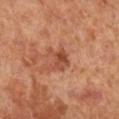Q: Is there a histopathology result?
A: no biopsy performed (imaged during a skin exam)
Q: Automated lesion metrics?
A: a footprint of about 5.5 mm², an eccentricity of roughly 0.5, and a shape-asymmetry score of about 0.45 (0 = symmetric); a nevus-likeness score of about 40/100 and a lesion-detection confidence of about 100/100
Q: Lesion location?
A: the right lower leg
Q: What is the imaging modality?
A: ~15 mm crop, total-body skin-cancer survey
Q: How was the tile lit?
A: cross-polarized illumination
Q: How large is the lesion?
A: ≈3 mm
Q: What are the patient's age and sex?
A: female, aged 63 to 67